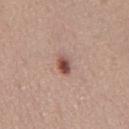Case summary:
- biopsy status: catalogued during a skin exam; not biopsied
- location: the mid back
- image: 15 mm crop, total-body photography
- lighting: white-light illumination
- subject: male, in their mid-50s
- lesion diameter: ~2.5 mm (longest diameter)